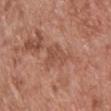Clinical impression: No biopsy was performed on this lesion — it was imaged during a full skin examination and was not determined to be concerning. Image and clinical context: The lesion is located on the chest. A lesion tile, about 15 mm wide, cut from a 3D total-body photograph. The lesion's longest dimension is about 4.5 mm. A male subject, roughly 70 years of age.